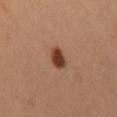| field | value |
|---|---|
| follow-up | no biopsy performed (imaged during a skin exam) |
| lesion size | ~3 mm (longest diameter) |
| TBP lesion metrics | an average lesion color of about L≈40 a*≈23 b*≈31 (CIELAB), roughly 15 lightness units darker than nearby skin, and a normalized border contrast of about 11.5; a nevus-likeness score of about 100/100 and a lesion-detection confidence of about 100/100 |
| lighting | cross-polarized |
| patient | male, approximately 50 years of age |
| anatomic site | the right thigh |
| acquisition | ~15 mm crop, total-body skin-cancer survey |